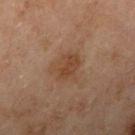Impression: No biopsy was performed on this lesion — it was imaged during a full skin examination and was not determined to be concerning. Clinical summary: The lesion is on the right upper arm. A 15 mm crop from a total-body photograph taken for skin-cancer surveillance. The subject is a male approximately 45 years of age.